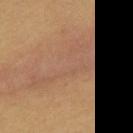– subject — female, aged approximately 25
– acquisition — ~15 mm crop, total-body skin-cancer survey
– body site — the upper back
– lesion diameter — ≈8.5 mm